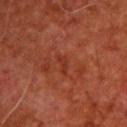Clinical summary:
This is a cross-polarized tile. A male subject aged approximately 60. Cropped from a total-body skin-imaging series; the visible field is about 15 mm. The lesion is on the upper back.
Conclusion:
Histopathologically confirmed as a malignant skin lesion: nodular basal cell carcinoma.